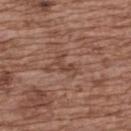Assessment:
No biopsy was performed on this lesion — it was imaged during a full skin examination and was not determined to be concerning.
Clinical summary:
The lesion-visualizer software estimated an average lesion color of about L≈43 a*≈20 b*≈27 (CIELAB), roughly 7 lightness units darker than nearby skin, and a normalized lesion–skin contrast near 6. It also reported a nevus-likeness score of about 0/100 and lesion-presence confidence of about 90/100. The recorded lesion diameter is about 3 mm. From the upper back. This is a white-light tile. A female subject aged 73 to 77. A 15 mm close-up extracted from a 3D total-body photography capture.